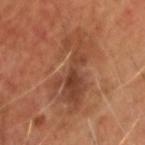This lesion was catalogued during total-body skin photography and was not selected for biopsy. The subject is a male about 65 years old. A roughly 15 mm field-of-view crop from a total-body skin photograph. The lesion's longest dimension is about 8.5 mm. Imaged with cross-polarized lighting. Located on the head or neck.